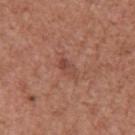notes: catalogued during a skin exam; not biopsied
lesion size: ≈3 mm
acquisition: 15 mm crop, total-body photography
patient: male, roughly 45 years of age
location: the left upper arm
illumination: white-light